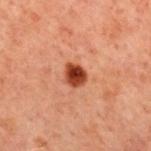Q: Was a biopsy performed?
A: catalogued during a skin exam; not biopsied
Q: How was this image acquired?
A: total-body-photography crop, ~15 mm field of view
Q: What lighting was used for the tile?
A: cross-polarized illumination
Q: Where on the body is the lesion?
A: the back
Q: Lesion size?
A: ~2.5 mm (longest diameter)
Q: What are the patient's age and sex?
A: male, about 60 years old